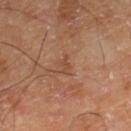biopsy status — no biopsy performed (imaged during a skin exam)
subject — male, aged 63 to 67
automated metrics — a mean CIELAB color near L≈48 a*≈22 b*≈32, a lesion–skin lightness drop of about 6, and a normalized lesion–skin contrast near 5; a color-variation rating of about 0/10 and a peripheral color-asymmetry measure near 0
body site — the right lower leg
acquisition — 15 mm crop, total-body photography